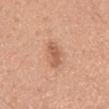Part of a total-body skin-imaging series; this lesion was reviewed on a skin check and was not flagged for biopsy.
A roughly 15 mm field-of-view crop from a total-body skin photograph.
The lesion is located on the mid back.
A male subject about 50 years old.
This is a white-light tile.
Longest diameter approximately 4 mm.
The total-body-photography lesion software estimated a shape eccentricity near 0.9 and a symmetry-axis asymmetry near 0.3. It also reported a normalized border contrast of about 6.5. And it measured a color-variation rating of about 2/10 and peripheral color asymmetry of about 0.5. And it measured a nevus-likeness score of about 45/100 and lesion-presence confidence of about 100/100.